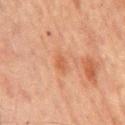follow-up — total-body-photography surveillance lesion; no biopsy | body site — the mid back | image-analysis metrics — a footprint of about 5.5 mm² and two-axis asymmetry of about 0.3; a within-lesion color-variation index near 2.5/10 and radial color variation of about 1; a nevus-likeness score of about 0/100 and lesion-presence confidence of about 100/100 | image — 15 mm crop, total-body photography | subject — male, aged 63–67.